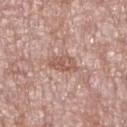<case>
<biopsy_status>not biopsied; imaged during a skin examination</biopsy_status>
<image>
  <source>total-body photography crop</source>
  <field_of_view_mm>15</field_of_view_mm>
</image>
<lesion_size>
  <long_diameter_mm_approx>3.5</long_diameter_mm_approx>
</lesion_size>
<patient>
  <sex>female</sex>
  <age_approx>75</age_approx>
</patient>
<site>right lower leg</site>
<lighting>white-light</lighting>
</case>